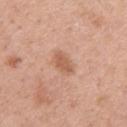Findings:
* site — the right upper arm
* subject — female, aged 38 to 42
* lighting — white-light
* image source — total-body-photography crop, ~15 mm field of view